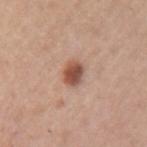Findings:
• follow-up — no biopsy performed (imaged during a skin exam)
• lighting — white-light
• diameter — about 2.5 mm
• body site — the left upper arm
• acquisition — ~15 mm tile from a whole-body skin photo
• patient — female, aged approximately 60
• image-analysis metrics — an area of roughly 5 mm² and a shape eccentricity near 0.55; border irregularity of about 1.5 on a 0–10 scale, a within-lesion color-variation index near 4.5/10, and peripheral color asymmetry of about 1.5; a detector confidence of about 100 out of 100 that the crop contains a lesion